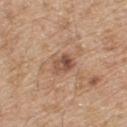Part of a total-body skin-imaging series; this lesion was reviewed on a skin check and was not flagged for biopsy.
A male patient, aged around 70.
The lesion is located on the upper back.
A close-up tile cropped from a whole-body skin photograph, about 15 mm across.
The recorded lesion diameter is about 2.5 mm.